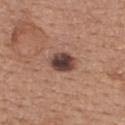{
  "biopsy_status": "not biopsied; imaged during a skin examination",
  "lighting": "white-light",
  "lesion_size": {
    "long_diameter_mm_approx": 3.0
  },
  "site": "back",
  "patient": {
    "sex": "female",
    "age_approx": 35
  },
  "automated_metrics": {
    "cielab_L": 41,
    "cielab_a": 19,
    "cielab_b": 22,
    "vs_skin_contrast_norm": 13.0,
    "nevus_likeness_0_100": 15,
    "lesion_detection_confidence_0_100": 100
  },
  "image": {
    "source": "total-body photography crop",
    "field_of_view_mm": 15
  }
}